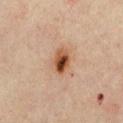Case summary:
• biopsy status · total-body-photography surveillance lesion; no biopsy
• anatomic site · the chest
• subject · male, aged approximately 45
• lesion size · ~3.5 mm (longest diameter)
• illumination · cross-polarized
• automated metrics · an area of roughly 6.5 mm²; a mean CIELAB color near L≈43 a*≈20 b*≈30, a lesion–skin lightness drop of about 13, and a normalized lesion–skin contrast near 11; a classifier nevus-likeness of about 100/100
• image · total-body-photography crop, ~15 mm field of view